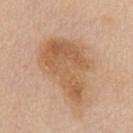* body site — the chest
* patient — female, aged 68 to 72
* imaging modality — ~15 mm crop, total-body skin-cancer survey
* lesion size — ≈8 mm
* lighting — white-light illumination
* image-analysis metrics — a shape-asymmetry score of about 0.4 (0 = symmetric); an automated nevus-likeness rating near 5 out of 100 and a detector confidence of about 100 out of 100 that the crop contains a lesion